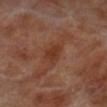Impression:
The lesion was photographed on a routine skin check and not biopsied; there is no pathology result.